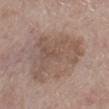TBP lesion metrics — an eccentricity of roughly 0.7 and two-axis asymmetry of about 0.45; a border-irregularity rating of about 7/10, a color-variation rating of about 3.5/10, and a peripheral color-asymmetry measure near 1; lesion-presence confidence of about 100/100 | illumination — white-light illumination | patient — female, about 65 years old | anatomic site — the right lower leg | image — ~15 mm crop, total-body skin-cancer survey.